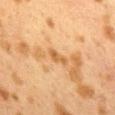Part of a total-body skin-imaging series; this lesion was reviewed on a skin check and was not flagged for biopsy. A 15 mm close-up tile from a total-body photography series done for melanoma screening. The recorded lesion diameter is about 3 mm. From the back. The patient is a female approximately 40 years of age. The total-body-photography lesion software estimated a lesion area of about 2.5 mm² and a shape-asymmetry score of about 0.45 (0 = symmetric). Imaged with cross-polarized lighting.